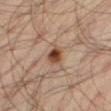Image and clinical context: From the leg. This is a cross-polarized tile. A 15 mm close-up extracted from a 3D total-body photography capture. A male subject, in their 50s.